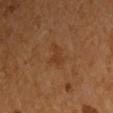This lesion was catalogued during total-body skin photography and was not selected for biopsy. Located on the right upper arm. A 15 mm crop from a total-body photograph taken for skin-cancer surveillance. About 2.5 mm across. The lesion-visualizer software estimated border irregularity of about 6 on a 0–10 scale and a within-lesion color-variation index near 0.5/10. The subject is a male aged around 55. Imaged with cross-polarized lighting.